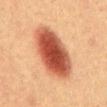Impression: Imaged during a routine full-body skin examination; the lesion was not biopsied and no histopathology is available. Clinical summary: Located on the front of the torso. A 15 mm close-up extracted from a 3D total-body photography capture. The subject is a male roughly 40 years of age. The recorded lesion diameter is about 7.5 mm.